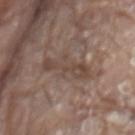notes: no biopsy performed (imaged during a skin exam); body site: the chest; acquisition: ~15 mm tile from a whole-body skin photo; subject: male, aged approximately 80.